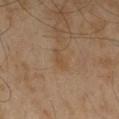Q: Was this lesion biopsied?
A: catalogued during a skin exam; not biopsied
Q: How was this image acquired?
A: 15 mm crop, total-body photography
Q: Where on the body is the lesion?
A: the left thigh
Q: Who is the patient?
A: male, roughly 60 years of age
Q: How was the tile lit?
A: cross-polarized illumination
Q: Automated lesion metrics?
A: an area of roughly 3 mm², an outline eccentricity of about 0.85 (0 = round, 1 = elongated), and a symmetry-axis asymmetry near 0.35; a border-irregularity index near 4/10 and a color-variation rating of about 0/10
Q: Lesion size?
A: ≈2.5 mm